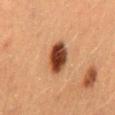Findings:
* workup: total-body-photography surveillance lesion; no biopsy
* patient: female, in their mid- to late 50s
* location: the mid back
* image: total-body-photography crop, ~15 mm field of view